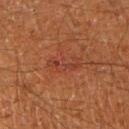Notes:
• follow-up · no biopsy performed (imaged during a skin exam)
• subject · male, roughly 65 years of age
• anatomic site · the right upper arm
• acquisition · total-body-photography crop, ~15 mm field of view